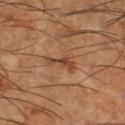Recorded during total-body skin imaging; not selected for excision or biopsy. Located on the right lower leg. A male patient roughly 65 years of age. Longest diameter approximately 3.5 mm. This image is a 15 mm lesion crop taken from a total-body photograph. The lesion-visualizer software estimated an average lesion color of about L≈45 a*≈21 b*≈33 (CIELAB) and a normalized lesion–skin contrast near 7. It also reported a border-irregularity rating of about 6.5/10 and a peripheral color-asymmetry measure near 0. It also reported a nevus-likeness score of about 0/100 and a detector confidence of about 95 out of 100 that the crop contains a lesion.